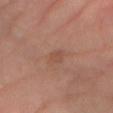notes: catalogued during a skin exam; not biopsied | site: the right forearm | subject: female, aged 63 to 67 | image source: ~15 mm tile from a whole-body skin photo.